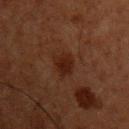follow-up: imaged on a skin check; not biopsied | tile lighting: cross-polarized | image: total-body-photography crop, ~15 mm field of view | automated lesion analysis: a border-irregularity index near 2.5/10 and a color-variation rating of about 2/10; a nevus-likeness score of about 35/100 | diameter: ≈3 mm | subject: male, aged 58–62 | anatomic site: the chest.